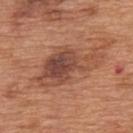Impression:
This lesion was catalogued during total-body skin photography and was not selected for biopsy.
Clinical summary:
Automated image analysis of the tile measured an area of roughly 22 mm², an eccentricity of roughly 0.85, and two-axis asymmetry of about 0.4. It also reported an average lesion color of about L≈48 a*≈23 b*≈30 (CIELAB), a lesion–skin lightness drop of about 11, and a normalized lesion–skin contrast near 8. It also reported a border-irregularity rating of about 6/10, a color-variation rating of about 8/10, and a peripheral color-asymmetry measure near 2.5. The software also gave an automated nevus-likeness rating near 15 out of 100. A male patient in their mid- to late 60s. A 15 mm close-up tile from a total-body photography series done for melanoma screening. Captured under white-light illumination. Located on the upper back.